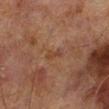Recorded during total-body skin imaging; not selected for excision or biopsy. This is a cross-polarized tile. A 15 mm crop from a total-body photograph taken for skin-cancer surveillance. Longest diameter approximately 3 mm. A male subject, aged 68 to 72. From the left lower leg. Automated image analysis of the tile measured border irregularity of about 4 on a 0–10 scale and a color-variation rating of about 0/10.